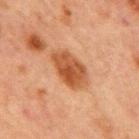  biopsy_status: not biopsied; imaged during a skin examination
  image:
    source: total-body photography crop
    field_of_view_mm: 15
  lighting: cross-polarized
  lesion_size:
    long_diameter_mm_approx: 5.5
  site: chest
  patient:
    sex: male
    age_approx: 65
  automated_metrics:
    area_mm2_approx: 13.0
    eccentricity: 0.85
    shape_asymmetry: 0.15